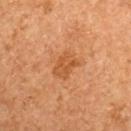follow-up: imaged on a skin check; not biopsied
site: the back
subject: female, approximately 60 years of age
imaging modality: total-body-photography crop, ~15 mm field of view